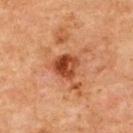Captured during whole-body skin photography for melanoma surveillance; the lesion was not biopsied.
A female subject, approximately 60 years of age.
Approximately 3 mm at its widest.
On the upper back.
This is a cross-polarized tile.
A 15 mm crop from a total-body photograph taken for skin-cancer surveillance.